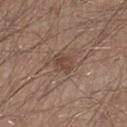Clinical impression: Imaged during a routine full-body skin examination; the lesion was not biopsied and no histopathology is available. Context: A 15 mm close-up tile from a total-body photography series done for melanoma screening. A male subject, in their 30s. Imaged with white-light lighting. From the right lower leg. An algorithmic analysis of the crop reported an area of roughly 5 mm², an eccentricity of roughly 0.55, and a shape-asymmetry score of about 0.2 (0 = symmetric). It also reported a lesion color around L≈44 a*≈17 b*≈25 in CIELAB, roughly 8 lightness units darker than nearby skin, and a lesion-to-skin contrast of about 6.5 (normalized; higher = more distinct). The analysis additionally found a within-lesion color-variation index near 2.5/10 and radial color variation of about 1. Longest diameter approximately 2.5 mm.